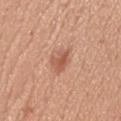Imaged during a routine full-body skin examination; the lesion was not biopsied and no histopathology is available. Measured at roughly 4 mm in maximum diameter. The lesion-visualizer software estimated an area of roughly 6 mm² and a symmetry-axis asymmetry near 0.3. The analysis additionally found a nevus-likeness score of about 70/100 and a detector confidence of about 100 out of 100 that the crop contains a lesion. A 15 mm close-up tile from a total-body photography series done for melanoma screening. A male patient roughly 30 years of age. Captured under white-light illumination. The lesion is on the head or neck.